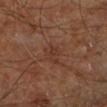workup=imaged on a skin check; not biopsied | subject=in their mid- to late 60s | TBP lesion metrics=an eccentricity of roughly 0.75; an average lesion color of about L≈34 a*≈21 b*≈26 (CIELAB), a lesion–skin lightness drop of about 5, and a normalized border contrast of about 5; an automated nevus-likeness rating near 0 out of 100 | illumination=cross-polarized illumination | diameter=about 3 mm | location=the left lower leg | acquisition=total-body-photography crop, ~15 mm field of view.